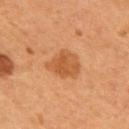Assessment: The lesion was tiled from a total-body skin photograph and was not biopsied. Clinical summary: The recorded lesion diameter is about 4 mm. Cropped from a total-body skin-imaging series; the visible field is about 15 mm. A male subject, aged 53 to 57. From the back. Imaged with cross-polarized lighting.